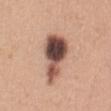Impression: The lesion was tiled from a total-body skin photograph and was not biopsied. Image and clinical context: On the chest. Approximately 7 mm at its widest. Imaged with white-light lighting. This image is a 15 mm lesion crop taken from a total-body photograph. The lesion-visualizer software estimated a lesion area of about 17 mm², a shape eccentricity near 0.85, and two-axis asymmetry of about 0.4. It also reported a mean CIELAB color near L≈50 a*≈20 b*≈25 and roughly 20 lightness units darker than nearby skin. And it measured border irregularity of about 4 on a 0–10 scale and radial color variation of about 4. The patient is a female in their 30s.